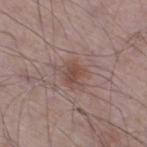image: 15 mm crop, total-body photography | patient: male, roughly 65 years of age | illumination: white-light | anatomic site: the right thigh | size: about 2.5 mm.